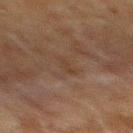Q: Was a biopsy performed?
A: imaged on a skin check; not biopsied
Q: What is the anatomic site?
A: the mid back
Q: Illumination type?
A: cross-polarized
Q: What is the imaging modality?
A: ~15 mm tile from a whole-body skin photo
Q: What is the lesion's diameter?
A: about 2.5 mm
Q: Patient demographics?
A: male, about 75 years old
Q: What did automated image analysis measure?
A: about 4 CIELAB-L* units darker than the surrounding skin and a lesion-to-skin contrast of about 5 (normalized; higher = more distinct)